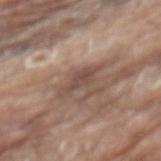Notes:
* imaging modality — 15 mm crop, total-body photography
* subject — male, approximately 80 years of age
* lighting — white-light
* anatomic site — the mid back